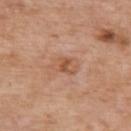{"automated_metrics": {"area_mm2_approx": 6.0, "eccentricity": 0.85, "shape_asymmetry": 0.2, "border_irregularity_0_10": 2.0, "color_variation_0_10": 5.0, "peripheral_color_asymmetry": 1.5, "nevus_likeness_0_100": 0, "lesion_detection_confidence_0_100": 100}, "lesion_size": {"long_diameter_mm_approx": 3.5}, "image": {"source": "total-body photography crop", "field_of_view_mm": 15}, "lighting": "white-light", "site": "back", "patient": {"sex": "male", "age_approx": 60}}